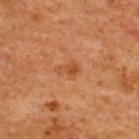This lesion was catalogued during total-body skin photography and was not selected for biopsy.
This image is a 15 mm lesion crop taken from a total-body photograph.
The lesion is located on the upper back.
The tile uses cross-polarized illumination.
Measured at roughly 2.5 mm in maximum diameter.
A male patient, aged around 65.